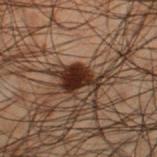follow-up: total-body-photography surveillance lesion; no biopsy
patient: male, approximately 50 years of age
site: the left thigh
image source: 15 mm crop, total-body photography
illumination: cross-polarized